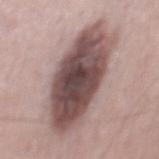This lesion was catalogued during total-body skin photography and was not selected for biopsy.
The subject is a male aged 48 to 52.
A 15 mm crop from a total-body photograph taken for skin-cancer surveillance.
An algorithmic analysis of the crop reported a lesion area of about 45 mm² and a shape eccentricity near 0.9. The software also gave a lesion color around L≈49 a*≈18 b*≈18 in CIELAB, about 19 CIELAB-L* units darker than the surrounding skin, and a lesion-to-skin contrast of about 12.5 (normalized; higher = more distinct). The software also gave a border-irregularity rating of about 2.5/10, a within-lesion color-variation index near 8/10, and peripheral color asymmetry of about 2.5. It also reported lesion-presence confidence of about 95/100.
On the mid back.